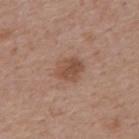The lesion is located on the upper back. The lesion-visualizer software estimated a footprint of about 7 mm², a shape eccentricity near 0.5, and a symmetry-axis asymmetry near 0.2. The software also gave a nevus-likeness score of about 45/100 and lesion-presence confidence of about 100/100. A male subject, aged around 60. A 15 mm close-up tile from a total-body photography series done for melanoma screening. The recorded lesion diameter is about 3 mm.